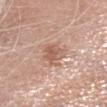The lesion was tiled from a total-body skin photograph and was not biopsied.
A female subject about 75 years old.
Cropped from a total-body skin-imaging series; the visible field is about 15 mm.
The lesion is on the head or neck.
Automated image analysis of the tile measured an automated nevus-likeness rating near 0 out of 100 and a detector confidence of about 100 out of 100 that the crop contains a lesion.
Captured under white-light illumination.
Longest diameter approximately 3.5 mm.